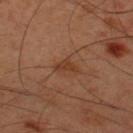  biopsy_status: not biopsied; imaged during a skin examination
  patient:
    sex: male
    age_approx: 45
  site: upper back
  image:
    source: total-body photography crop
    field_of_view_mm: 15